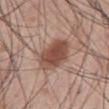Clinical summary: A male patient roughly 45 years of age. Automated tile analysis of the lesion measured about 13 CIELAB-L* units darker than the surrounding skin and a normalized border contrast of about 9.5. The analysis additionally found a border-irregularity rating of about 1.5/10 and a color-variation rating of about 4/10. It also reported a classifier nevus-likeness of about 95/100 and a detector confidence of about 100 out of 100 that the crop contains a lesion. A 15 mm close-up extracted from a 3D total-body photography capture. The lesion is located on the front of the torso. The recorded lesion diameter is about 5 mm. Imaged with white-light lighting.